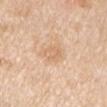Part of a total-body skin-imaging series; this lesion was reviewed on a skin check and was not flagged for biopsy. About 2.5 mm across. A region of skin cropped from a whole-body photographic capture, roughly 15 mm wide. Located on the right upper arm. Automated image analysis of the tile measured a footprint of about 4.5 mm². It also reported an average lesion color of about L≈68 a*≈19 b*≈35 (CIELAB), roughly 7 lightness units darker than nearby skin, and a normalized lesion–skin contrast near 4.5. And it measured a classifier nevus-likeness of about 0/100 and lesion-presence confidence of about 100/100. A male subject aged 58–62.